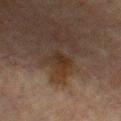Captured during whole-body skin photography for melanoma surveillance; the lesion was not biopsied. A region of skin cropped from a whole-body photographic capture, roughly 15 mm wide. This is a cross-polarized tile. The lesion is located on the upper back. About 2.5 mm across. The patient is a male in their mid- to late 60s.